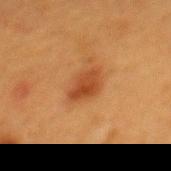From the mid back.
The lesion's longest dimension is about 4 mm.
Imaged with cross-polarized lighting.
Cropped from a whole-body photographic skin survey; the tile spans about 15 mm.
A female subject, aged 38–42.